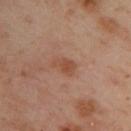The lesion was tiled from a total-body skin photograph and was not biopsied. Cropped from a total-body skin-imaging series; the visible field is about 15 mm. The recorded lesion diameter is about 3 mm. The subject is a female aged 38–42. The lesion is located on the upper back.